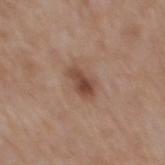Q: Was a biopsy performed?
A: catalogued during a skin exam; not biopsied
Q: What are the patient's age and sex?
A: male, aged approximately 60
Q: What kind of image is this?
A: ~15 mm crop, total-body skin-cancer survey
Q: Lesion size?
A: ~3 mm (longest diameter)
Q: What lighting was used for the tile?
A: white-light illumination
Q: Lesion location?
A: the mid back
Q: Automated lesion metrics?
A: a footprint of about 5.5 mm², a shape eccentricity near 0.8, and two-axis asymmetry of about 0.25; radial color variation of about 1.5